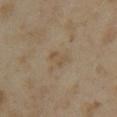diameter = ≈3 mm | site = the left upper arm | image = total-body-photography crop, ~15 mm field of view | patient = female, aged 33–37 | automated lesion analysis = a mean CIELAB color near L≈48 a*≈11 b*≈29, a lesion–skin lightness drop of about 5, and a normalized border contrast of about 5; a border-irregularity rating of about 4/10, a within-lesion color-variation index near 1.5/10, and peripheral color asymmetry of about 0.5 | tile lighting = cross-polarized.